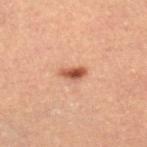workup: no biopsy performed (imaged during a skin exam)
site: the right lower leg
image: total-body-photography crop, ~15 mm field of view
subject: female, in their mid-40s